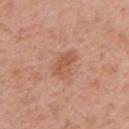biopsy status — imaged on a skin check; not biopsied | body site — the right upper arm | acquisition — 15 mm crop, total-body photography | patient — female, roughly 45 years of age | lesion size — ≈3.5 mm | tile lighting — white-light | automated metrics — an area of roughly 6.5 mm², a shape eccentricity near 0.75, and a symmetry-axis asymmetry near 0.3; an automated nevus-likeness rating near 0 out of 100 and a lesion-detection confidence of about 100/100.